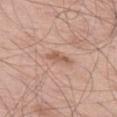Clinical impression:
Imaged during a routine full-body skin examination; the lesion was not biopsied and no histopathology is available.
Image and clinical context:
A male subject aged 68 to 72. A lesion tile, about 15 mm wide, cut from a 3D total-body photograph. On the left thigh.